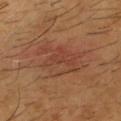Clinical impression:
The lesion was tiled from a total-body skin photograph and was not biopsied.